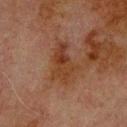biopsy_status: not biopsied; imaged during a skin examination
lighting: cross-polarized
lesion_size:
  long_diameter_mm_approx: 5.0
site: back
patient:
  sex: male
  age_approx: 80
image:
  source: total-body photography crop
  field_of_view_mm: 15